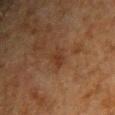Q: Was a biopsy performed?
A: no biopsy performed (imaged during a skin exam)
Q: What is the lesion's diameter?
A: ≈2.5 mm
Q: Where on the body is the lesion?
A: the chest
Q: What is the imaging modality?
A: total-body-photography crop, ~15 mm field of view
Q: Illumination type?
A: cross-polarized
Q: What are the patient's age and sex?
A: male, roughly 50 years of age